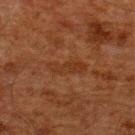No biopsy was performed on this lesion — it was imaged during a full skin examination and was not determined to be concerning. The lesion is located on the upper back. The lesion's longest dimension is about 4.5 mm. A lesion tile, about 15 mm wide, cut from a 3D total-body photograph. Captured under cross-polarized illumination. The patient is a male aged approximately 60.